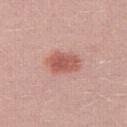Clinical impression: This lesion was catalogued during total-body skin photography and was not selected for biopsy. Acquisition and patient details: A close-up tile cropped from a whole-body skin photograph, about 15 mm across. The total-body-photography lesion software estimated a lesion area of about 9.5 mm², a shape eccentricity near 0.75, and a shape-asymmetry score of about 0.15 (0 = symmetric). And it measured internal color variation of about 3 on a 0–10 scale and a peripheral color-asymmetry measure near 1. Approximately 4 mm at its widest. The lesion is on the right thigh. This is a white-light tile. A female patient about 25 years old.